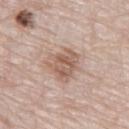This lesion was catalogued during total-body skin photography and was not selected for biopsy.
Automated tile analysis of the lesion measured an area of roughly 11 mm² and an eccentricity of roughly 0.55. The software also gave a lesion color around L≈58 a*≈18 b*≈27 in CIELAB and a normalized lesion–skin contrast near 7. The software also gave a nevus-likeness score of about 10/100 and a lesion-detection confidence of about 100/100.
From the left thigh.
A male patient aged approximately 80.
Imaged with white-light lighting.
The recorded lesion diameter is about 4 mm.
A lesion tile, about 15 mm wide, cut from a 3D total-body photograph.